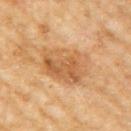Q: Was a biopsy performed?
A: imaged on a skin check; not biopsied
Q: How was this image acquired?
A: ~15 mm crop, total-body skin-cancer survey
Q: What is the lesion's diameter?
A: about 6 mm
Q: What is the anatomic site?
A: the right upper arm
Q: Who is the patient?
A: female, aged 58–62